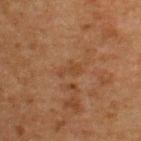{"biopsy_status": "not biopsied; imaged during a skin examination", "lesion_size": {"long_diameter_mm_approx": 2.5}, "patient": {"sex": "male", "age_approx": 50}, "automated_metrics": {"cielab_L": 35, "cielab_a": 18, "cielab_b": 29, "vs_skin_darker_L": 5.0, "peripheral_color_asymmetry": 0.0, "nevus_likeness_0_100": 0}, "image": {"source": "total-body photography crop", "field_of_view_mm": 15}, "lighting": "cross-polarized", "site": "upper back"}